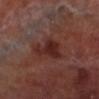Q: Was a biopsy performed?
A: imaged on a skin check; not biopsied
Q: What are the patient's age and sex?
A: male, approximately 70 years of age
Q: What kind of image is this?
A: 15 mm crop, total-body photography
Q: What is the anatomic site?
A: the left lower leg